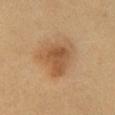Impression: Captured during whole-body skin photography for melanoma surveillance; the lesion was not biopsied. Image and clinical context: A lesion tile, about 15 mm wide, cut from a 3D total-body photograph. The subject is a male about 60 years old. An algorithmic analysis of the crop reported a mean CIELAB color near L≈51 a*≈19 b*≈35, roughly 10 lightness units darker than nearby skin, and a normalized border contrast of about 7.5. The software also gave a border-irregularity rating of about 4/10, internal color variation of about 4.5 on a 0–10 scale, and radial color variation of about 1.5. The analysis additionally found an automated nevus-likeness rating near 85 out of 100 and a detector confidence of about 100 out of 100 that the crop contains a lesion. The lesion is located on the front of the torso. Captured under cross-polarized illumination. Approximately 5.5 mm at its widest.